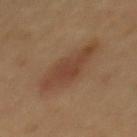Recorded during total-body skin imaging; not selected for excision or biopsy. The tile uses cross-polarized illumination. On the mid back. About 7.5 mm across. A male subject, aged around 50. A 15 mm close-up extracted from a 3D total-body photography capture.